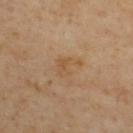follow-up — total-body-photography surveillance lesion; no biopsy | acquisition — ~15 mm crop, total-body skin-cancer survey | location — the upper back | tile lighting — cross-polarized illumination | size — ~3 mm (longest diameter) | TBP lesion metrics — a lesion area of about 4.5 mm², an outline eccentricity of about 0.7 (0 = round, 1 = elongated), and a shape-asymmetry score of about 0.5 (0 = symmetric); a border-irregularity rating of about 6/10, internal color variation of about 2 on a 0–10 scale, and a peripheral color-asymmetry measure near 0.5 | subject — male, aged 53 to 57.